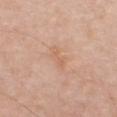notes = imaged on a skin check; not biopsied | illumination = white-light | patient = male, aged around 70 | automated lesion analysis = a footprint of about 4.5 mm², a shape eccentricity near 0.85, and two-axis asymmetry of about 0.35; a border-irregularity rating of about 3.5/10, a within-lesion color-variation index near 1.5/10, and a peripheral color-asymmetry measure near 0.5; a nevus-likeness score of about 0/100 and a detector confidence of about 100 out of 100 that the crop contains a lesion | imaging modality = total-body-photography crop, ~15 mm field of view | location = the front of the torso.